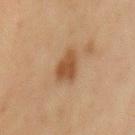| feature | finding |
|---|---|
| biopsy status | no biopsy performed (imaged during a skin exam) |
| automated lesion analysis | about 10 CIELAB-L* units darker than the surrounding skin and a normalized border contrast of about 8.5; a color-variation rating of about 2/10 |
| subject | male, aged 63–67 |
| illumination | cross-polarized illumination |
| location | the mid back |
| acquisition | ~15 mm tile from a whole-body skin photo |
| diameter | about 3 mm |